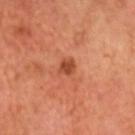  biopsy_status: not biopsied; imaged during a skin examination